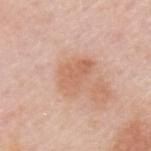Clinical impression:
Recorded during total-body skin imaging; not selected for excision or biopsy.
Image and clinical context:
The lesion's longest dimension is about 4.5 mm. A male patient approximately 60 years of age. On the upper back. An algorithmic analysis of the crop reported a lesion area of about 9.5 mm², an outline eccentricity of about 0.75 (0 = round, 1 = elongated), and a symmetry-axis asymmetry near 0.35. It also reported a mean CIELAB color near L≈63 a*≈22 b*≈32, a lesion–skin lightness drop of about 8, and a normalized lesion–skin contrast near 6. And it measured a lesion-detection confidence of about 100/100. A close-up tile cropped from a whole-body skin photograph, about 15 mm across. This is a white-light tile.